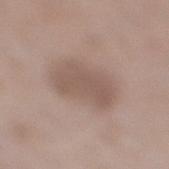Findings:
– notes: total-body-photography surveillance lesion; no biopsy
– tile lighting: white-light illumination
– site: the right lower leg
– image: ~15 mm crop, total-body skin-cancer survey
– diameter: ≈5 mm
– patient: male, about 70 years old